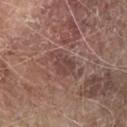Acquisition and patient details:
A roughly 15 mm field-of-view crop from a total-body skin photograph. The recorded lesion diameter is about 3.5 mm. Located on the right forearm. A male patient approximately 80 years of age. An algorithmic analysis of the crop reported a footprint of about 6 mm² and two-axis asymmetry of about 0.4.